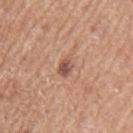workup: no biopsy performed (imaged during a skin exam) | lesion size: ~3 mm (longest diameter) | site: the left upper arm | acquisition: 15 mm crop, total-body photography | TBP lesion metrics: an outline eccentricity of about 0.7 (0 = round, 1 = elongated) and two-axis asymmetry of about 0.3; an average lesion color of about L≈55 a*≈21 b*≈28 (CIELAB) and a normalized border contrast of about 7; a border-irregularity index near 2.5/10 and internal color variation of about 5 on a 0–10 scale; a nevus-likeness score of about 85/100 and a lesion-detection confidence of about 100/100 | lighting: white-light | patient: male, aged 73 to 77.